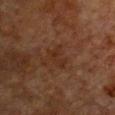Case summary:
* biopsy status: total-body-photography surveillance lesion; no biopsy
* body site: the chest
* image source: 15 mm crop, total-body photography
* subject: female, roughly 55 years of age
* lesion diameter: ≈3 mm